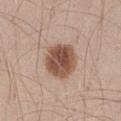workup: total-body-photography surveillance lesion; no biopsy
size: ~4.5 mm (longest diameter)
body site: the right thigh
automated lesion analysis: a footprint of about 15 mm², an outline eccentricity of about 0.3 (0 = round, 1 = elongated), and a shape-asymmetry score of about 0.1 (0 = symmetric); a border-irregularity rating of about 1/10, a within-lesion color-variation index near 6/10, and radial color variation of about 2; a classifier nevus-likeness of about 100/100 and a detector confidence of about 100 out of 100 that the crop contains a lesion
illumination: white-light
subject: male, in their 30s
image source: 15 mm crop, total-body photography